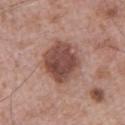biopsy status: imaged on a skin check; not biopsied
automated lesion analysis: border irregularity of about 1.5 on a 0–10 scale and radial color variation of about 1.5; an automated nevus-likeness rating near 25 out of 100
image: ~15 mm crop, total-body skin-cancer survey
subject: male, approximately 65 years of age
lighting: white-light
body site: the left upper arm
lesion size: about 5 mm